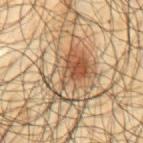follow-up: imaged on a skin check; not biopsied
body site: the mid back
diameter: about 8.5 mm
subject: male, aged around 65
illumination: cross-polarized
acquisition: 15 mm crop, total-body photography